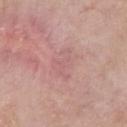Case summary:
• biopsy status: catalogued during a skin exam; not biopsied
• patient: female, approximately 70 years of age
• illumination: white-light illumination
• body site: the chest
• automated metrics: a footprint of about 9.5 mm² and an outline eccentricity of about 0.7 (0 = round, 1 = elongated); a mean CIELAB color near L≈61 a*≈23 b*≈21, roughly 5 lightness units darker than nearby skin, and a normalized lesion–skin contrast near 3; a lesion-detection confidence of about 90/100
• acquisition: 15 mm crop, total-body photography
• lesion diameter: ≈4 mm